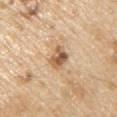{
  "biopsy_status": "not biopsied; imaged during a skin examination",
  "lesion_size": {
    "long_diameter_mm_approx": 3.0
  },
  "automated_metrics": {
    "area_mm2_approx": 6.0,
    "eccentricity": 0.75,
    "shape_asymmetry": 0.25,
    "border_irregularity_0_10": 2.5,
    "peripheral_color_asymmetry": 2.0
  },
  "site": "left upper arm",
  "patient": {
    "sex": "male",
    "age_approx": 70
  },
  "image": {
    "source": "total-body photography crop",
    "field_of_view_mm": 15
  }
}